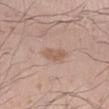Clinical impression: The lesion was photographed on a routine skin check and not biopsied; there is no pathology result. Acquisition and patient details: Imaged with white-light lighting. Cropped from a whole-body photographic skin survey; the tile spans about 15 mm. Automated image analysis of the tile measured a footprint of about 5 mm² and a shape-asymmetry score of about 0.25 (0 = symmetric). A male subject in their mid-20s. Longest diameter approximately 4 mm. The lesion is located on the left lower leg.